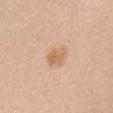Q: Is there a histopathology result?
A: imaged on a skin check; not biopsied
Q: Illumination type?
A: white-light
Q: Patient demographics?
A: female, aged 48 to 52
Q: Lesion location?
A: the chest
Q: How was this image acquired?
A: ~15 mm tile from a whole-body skin photo
Q: Lesion size?
A: ~3 mm (longest diameter)